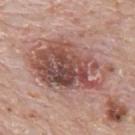follow-up: total-body-photography surveillance lesion; no biopsy
body site: the mid back
imaging modality: total-body-photography crop, ~15 mm field of view
patient: male, about 80 years old
lesion diameter: ~9 mm (longest diameter)
automated lesion analysis: an outline eccentricity of about 0.8 (0 = round, 1 = elongated) and a symmetry-axis asymmetry near 0.2; a lesion color around L≈51 a*≈22 b*≈24 in CIELAB, a lesion–skin lightness drop of about 14, and a lesion-to-skin contrast of about 9.5 (normalized; higher = more distinct); a nevus-likeness score of about 5/100 and lesion-presence confidence of about 100/100